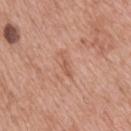Clinical summary:
The tile uses white-light illumination. Longest diameter approximately 3 mm. The lesion is on the upper back. The patient is a male about 75 years old. A 15 mm close-up tile from a total-body photography series done for melanoma screening. Automated image analysis of the tile measured an area of roughly 3 mm² and a symmetry-axis asymmetry near 0.4. It also reported a mean CIELAB color near L≈57 a*≈23 b*≈31, roughly 7 lightness units darker than nearby skin, and a normalized border contrast of about 5.5. It also reported a border-irregularity rating of about 4.5/10, a color-variation rating of about 0.5/10, and a peripheral color-asymmetry measure near 0. The analysis additionally found an automated nevus-likeness rating near 0 out of 100 and lesion-presence confidence of about 100/100.